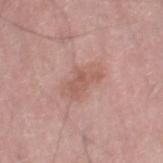Assessment: The lesion was photographed on a routine skin check and not biopsied; there is no pathology result. Background: Located on the right thigh. Captured under white-light illumination. A male patient, roughly 60 years of age. A lesion tile, about 15 mm wide, cut from a 3D total-body photograph.